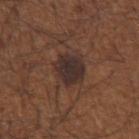Impression:
Part of a total-body skin-imaging series; this lesion was reviewed on a skin check and was not flagged for biopsy.
Context:
Captured under white-light illumination. On the arm. A male patient in their mid- to late 60s. A roughly 15 mm field-of-view crop from a total-body skin photograph.